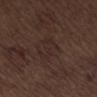Case summary:
- workup: catalogued during a skin exam; not biopsied
- patient: male, approximately 70 years of age
- size: ≈4 mm
- tile lighting: white-light illumination
- acquisition: ~15 mm crop, total-body skin-cancer survey
- automated lesion analysis: a footprint of about 6 mm² and a symmetry-axis asymmetry near 0.5; a mean CIELAB color near L≈25 a*≈15 b*≈17 and a normalized lesion–skin contrast near 5; a nevus-likeness score of about 0/100 and a lesion-detection confidence of about 70/100
- anatomic site: the abdomen